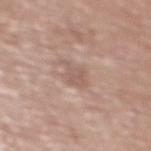workup: imaged on a skin check; not biopsied
image-analysis metrics: a lesion color around L≈57 a*≈18 b*≈25 in CIELAB and a lesion–skin lightness drop of about 7; border irregularity of about 4.5 on a 0–10 scale and peripheral color asymmetry of about 0.5
lighting: white-light illumination
location: the upper back
image source: 15 mm crop, total-body photography
patient: female, in their 70s